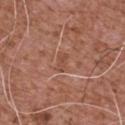<lesion>
<biopsy_status>not biopsied; imaged during a skin examination</biopsy_status>
<image>
  <source>total-body photography crop</source>
  <field_of_view_mm>15</field_of_view_mm>
</image>
<patient>
  <sex>male</sex>
  <age_approx>75</age_approx>
</patient>
<site>front of the torso</site>
</lesion>